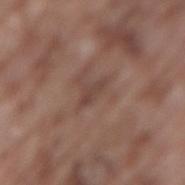Recorded during total-body skin imaging; not selected for excision or biopsy. Cropped from a total-body skin-imaging series; the visible field is about 15 mm. A male patient approximately 75 years of age. The lesion is located on the lower back.